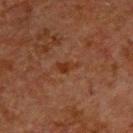Q: Was a biopsy performed?
A: catalogued during a skin exam; not biopsied
Q: Automated lesion metrics?
A: a lesion area of about 3 mm², an eccentricity of roughly 0.85, and two-axis asymmetry of about 0.35; a mean CIELAB color near L≈26 a*≈19 b*≈27; internal color variation of about 1 on a 0–10 scale and radial color variation of about 0.5; a nevus-likeness score of about 0/100 and a detector confidence of about 100 out of 100 that the crop contains a lesion
Q: What are the patient's age and sex?
A: male, approximately 60 years of age
Q: How was this image acquired?
A: 15 mm crop, total-body photography
Q: Lesion location?
A: the back
Q: What lighting was used for the tile?
A: cross-polarized illumination